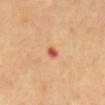Q: How was this image acquired?
A: 15 mm crop, total-body photography
Q: Where on the body is the lesion?
A: the mid back
Q: How was the tile lit?
A: cross-polarized
Q: What are the patient's age and sex?
A: male, aged 58 to 62